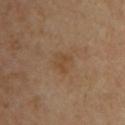This lesion was catalogued during total-body skin photography and was not selected for biopsy.
A male subject, aged 53–57.
About 2.5 mm across.
Imaged with cross-polarized lighting.
On the chest.
A lesion tile, about 15 mm wide, cut from a 3D total-body photograph.